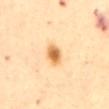{
  "biopsy_status": "not biopsied; imaged during a skin examination",
  "image": {
    "source": "total-body photography crop",
    "field_of_view_mm": 15
  },
  "lighting": "cross-polarized",
  "site": "abdomen",
  "patient": {
    "sex": "female",
    "age_approx": 65
  }
}